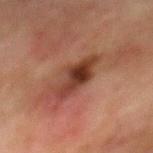Clinical impression: Part of a total-body skin-imaging series; this lesion was reviewed on a skin check and was not flagged for biopsy.